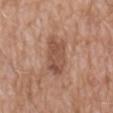Clinical impression:
The lesion was tiled from a total-body skin photograph and was not biopsied.
Acquisition and patient details:
The patient is a male aged approximately 60. A 15 mm crop from a total-body photograph taken for skin-cancer surveillance. An algorithmic analysis of the crop reported a footprint of about 11 mm² and an eccentricity of roughly 0.85. The analysis additionally found a mean CIELAB color near L≈51 a*≈21 b*≈29 and about 10 CIELAB-L* units darker than the surrounding skin. It also reported peripheral color asymmetry of about 1. From the abdomen. Approximately 5 mm at its widest.